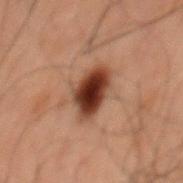Clinical impression: Recorded during total-body skin imaging; not selected for excision or biopsy. Background: A 15 mm crop from a total-body photograph taken for skin-cancer surveillance. The patient is a male aged 58 to 62. Located on the back.